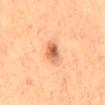Q: Was a biopsy performed?
A: imaged on a skin check; not biopsied
Q: What lighting was used for the tile?
A: cross-polarized
Q: Where on the body is the lesion?
A: the back
Q: What is the lesion's diameter?
A: about 3.5 mm
Q: Patient demographics?
A: female, aged around 60
Q: How was this image acquired?
A: total-body-photography crop, ~15 mm field of view
Q: Automated lesion metrics?
A: an average lesion color of about L≈60 a*≈26 b*≈36 (CIELAB) and a lesion-to-skin contrast of about 9 (normalized; higher = more distinct)